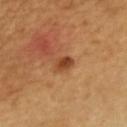Imaged during a routine full-body skin examination; the lesion was not biopsied and no histopathology is available. Imaged with cross-polarized lighting. A region of skin cropped from a whole-body photographic capture, roughly 15 mm wide. On the chest. Automated tile analysis of the lesion measured a footprint of about 4 mm² and an eccentricity of roughly 0.6. The software also gave peripheral color asymmetry of about 1. Longest diameter approximately 2.5 mm. A male patient in their mid- to late 40s.